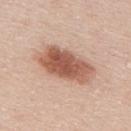Assessment: Recorded during total-body skin imaging; not selected for excision or biopsy. Image and clinical context: Measured at roughly 7 mm in maximum diameter. Automated image analysis of the tile measured a lesion area of about 19 mm², an eccentricity of roughly 0.85, and two-axis asymmetry of about 0.2. The analysis additionally found a lesion–skin lightness drop of about 16. The analysis additionally found border irregularity of about 2.5 on a 0–10 scale and a color-variation rating of about 5/10. The tile uses white-light illumination. The subject is a male in their 40s. A roughly 15 mm field-of-view crop from a total-body skin photograph. From the upper back.